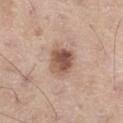Captured during whole-body skin photography for melanoma surveillance; the lesion was not biopsied.
Approximately 3.5 mm at its widest.
The lesion is on the right thigh.
A region of skin cropped from a whole-body photographic capture, roughly 15 mm wide.
Imaged with white-light lighting.
The patient is a male aged around 60.